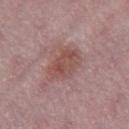| key | value |
|---|---|
| follow-up | catalogued during a skin exam; not biopsied |
| patient | female, in their mid- to late 40s |
| diameter | about 4.5 mm |
| location | the right thigh |
| illumination | white-light illumination |
| imaging modality | ~15 mm tile from a whole-body skin photo |
| automated metrics | border irregularity of about 3 on a 0–10 scale, internal color variation of about 4.5 on a 0–10 scale, and radial color variation of about 1.5; a classifier nevus-likeness of about 5/100 and a detector confidence of about 100 out of 100 that the crop contains a lesion |